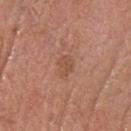follow-up: total-body-photography surveillance lesion; no biopsy.